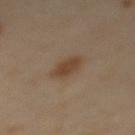Q: Who is the patient?
A: male, roughly 65 years of age
Q: What lighting was used for the tile?
A: cross-polarized
Q: What did automated image analysis measure?
A: a classifier nevus-likeness of about 95/100 and a detector confidence of about 100 out of 100 that the crop contains a lesion
Q: Where on the body is the lesion?
A: the mid back
Q: What is the lesion's diameter?
A: about 4 mm
Q: What is the imaging modality?
A: ~15 mm tile from a whole-body skin photo